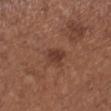Q: Was a biopsy performed?
A: total-body-photography surveillance lesion; no biopsy
Q: Lesion size?
A: ~2.5 mm (longest diameter)
Q: Lesion location?
A: the left lower leg
Q: Who is the patient?
A: female, about 55 years old
Q: How was this image acquired?
A: ~15 mm tile from a whole-body skin photo
Q: What lighting was used for the tile?
A: white-light